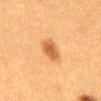Case summary:
– follow-up · catalogued during a skin exam; not biopsied
– imaging modality · ~15 mm tile from a whole-body skin photo
– TBP lesion metrics · a lesion color around L≈49 a*≈22 b*≈38 in CIELAB, a lesion–skin lightness drop of about 11, and a lesion-to-skin contrast of about 8 (normalized; higher = more distinct); a within-lesion color-variation index near 2.5/10 and peripheral color asymmetry of about 0.5
– site · the front of the torso
– lesion size · ~3 mm (longest diameter)
– patient · female, aged 38–42